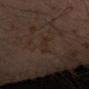Assessment:
Captured during whole-body skin photography for melanoma surveillance; the lesion was not biopsied.
Clinical summary:
The total-body-photography lesion software estimated two-axis asymmetry of about 0.3. The software also gave an average lesion color of about L≈22 a*≈12 b*≈19 (CIELAB) and roughly 3 lightness units darker than nearby skin. The software also gave border irregularity of about 4 on a 0–10 scale, a within-lesion color-variation index near 0/10, and peripheral color asymmetry of about 0. And it measured a nevus-likeness score of about 0/100 and a lesion-detection confidence of about 80/100. Cropped from a total-body skin-imaging series; the visible field is about 15 mm. Longest diameter approximately 3.5 mm. The patient is a male in their 60s. The tile uses cross-polarized illumination. The lesion is on the left forearm.